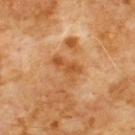The lesion was tiled from a total-body skin photograph and was not biopsied. A roughly 15 mm field-of-view crop from a total-body skin photograph. This is a cross-polarized tile. The lesion-visualizer software estimated a shape eccentricity near 0.85 and a shape-asymmetry score of about 0.35 (0 = symmetric). And it measured a lesion color around L≈51 a*≈25 b*≈41 in CIELAB. And it measured a border-irregularity rating of about 4/10, internal color variation of about 2.5 on a 0–10 scale, and peripheral color asymmetry of about 1. And it measured a nevus-likeness score of about 0/100 and a lesion-detection confidence of about 100/100. From the chest. A male patient, aged approximately 60. Approximately 3.5 mm at its widest.